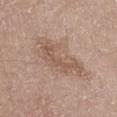Impression:
Imaged during a routine full-body skin examination; the lesion was not biopsied and no histopathology is available.
Background:
The lesion's longest dimension is about 6.5 mm. On the left thigh. This is a white-light tile. A lesion tile, about 15 mm wide, cut from a 3D total-body photograph. The subject is a male about 70 years old. The lesion-visualizer software estimated an average lesion color of about L≈56 a*≈17 b*≈27 (CIELAB), a lesion–skin lightness drop of about 8, and a lesion-to-skin contrast of about 6 (normalized; higher = more distinct). The software also gave a color-variation rating of about 2.5/10 and radial color variation of about 1. The software also gave lesion-presence confidence of about 100/100.